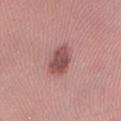This lesion was catalogued during total-body skin photography and was not selected for biopsy.
From the right forearm.
This is a white-light tile.
Automated tile analysis of the lesion measured a footprint of about 8 mm² and a symmetry-axis asymmetry near 0.25. The analysis additionally found a border-irregularity rating of about 2.5/10, internal color variation of about 3 on a 0–10 scale, and a peripheral color-asymmetry measure near 1. It also reported a nevus-likeness score of about 35/100 and a detector confidence of about 100 out of 100 that the crop contains a lesion.
The recorded lesion diameter is about 4 mm.
A close-up tile cropped from a whole-body skin photograph, about 15 mm across.
A female patient, approximately 20 years of age.